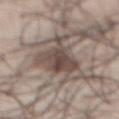{"image": {"source": "total-body photography crop", "field_of_view_mm": 15}, "lesion_size": {"long_diameter_mm_approx": 8.5}, "patient": {"sex": "male", "age_approx": 55}, "lighting": "white-light", "site": "front of the torso", "automated_metrics": {"area_mm2_approx": 21.0, "eccentricity": 0.85, "shape_asymmetry": 0.5, "border_irregularity_0_10": 7.0, "color_variation_0_10": 6.0, "peripheral_color_asymmetry": 2.0}}